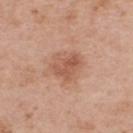Part of a total-body skin-imaging series; this lesion was reviewed on a skin check and was not flagged for biopsy. The lesion is located on the upper back. An algorithmic analysis of the crop reported an automated nevus-likeness rating near 5 out of 100 and a lesion-detection confidence of about 100/100. A male subject, aged approximately 65. This is a white-light tile. A 15 mm close-up tile from a total-body photography series done for melanoma screening.